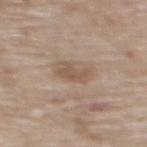<lesion>
<biopsy_status>not biopsied; imaged during a skin examination</biopsy_status>
<lesion_size>
  <long_diameter_mm_approx>3.0</long_diameter_mm_approx>
</lesion_size>
<automated_metrics>
  <shape_asymmetry>0.4</shape_asymmetry>
  <cielab_L>54</cielab_L>
  <cielab_a>15</cielab_a>
  <cielab_b>27</cielab_b>
  <vs_skin_darker_L>8.0</vs_skin_darker_L>
  <border_irregularity_0_10>4.0</border_irregularity_0_10>
  <color_variation_0_10>1.0</color_variation_0_10>
  <peripheral_color_asymmetry>0.5</peripheral_color_asymmetry>
  <nevus_likeness_0_100>0</nevus_likeness_0_100>
</automated_metrics>
<site>upper back</site>
<patient>
  <sex>female</sex>
  <age_approx>75</age_approx>
</patient>
<image>
  <source>total-body photography crop</source>
  <field_of_view_mm>15</field_of_view_mm>
</image>
<lighting>white-light</lighting>
</lesion>